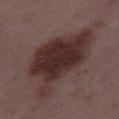<tbp_lesion>
  <biopsy_status>not biopsied; imaged during a skin examination</biopsy_status>
  <patient>
    <sex>female</sex>
    <age_approx>35</age_approx>
  </patient>
  <site>leg</site>
  <image>
    <source>total-body photography crop</source>
    <field_of_view_mm>15</field_of_view_mm>
  </image>
  <lesion_size>
    <long_diameter_mm_approx>10.5</long_diameter_mm_approx>
  </lesion_size>
  <lighting>white-light</lighting>
</tbp_lesion>